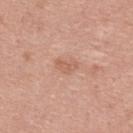A region of skin cropped from a whole-body photographic capture, roughly 15 mm wide. Approximately 2.5 mm at its widest. On the upper back. The patient is a female in their 40s. Captured under white-light illumination.